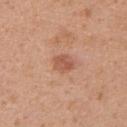Clinical impression:
This lesion was catalogued during total-body skin photography and was not selected for biopsy.
Image and clinical context:
A lesion tile, about 15 mm wide, cut from a 3D total-body photograph. A female subject, aged around 25. From the upper back.